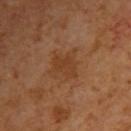Image and clinical context:
The lesion is on the upper back. Automated tile analysis of the lesion measured a lesion area of about 5.5 mm², an eccentricity of roughly 0.7, and two-axis asymmetry of about 0.35. The analysis additionally found a border-irregularity rating of about 3.5/10 and internal color variation of about 2 on a 0–10 scale. And it measured a nevus-likeness score of about 0/100 and lesion-presence confidence of about 100/100. The patient is a female roughly 40 years of age. A 15 mm close-up tile from a total-body photography series done for melanoma screening. Longest diameter approximately 3 mm. Imaged with cross-polarized lighting.